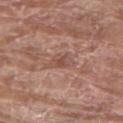Recorded during total-body skin imaging; not selected for excision or biopsy. The tile uses white-light illumination. From the chest. A male patient aged around 80. Cropped from a total-body skin-imaging series; the visible field is about 15 mm. About 3.5 mm across.